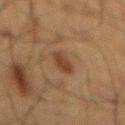notes: total-body-photography surveillance lesion; no biopsy.